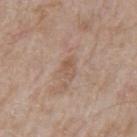biopsy_status: not biopsied; imaged during a skin examination
site: mid back
image:
  source: total-body photography crop
  field_of_view_mm: 15
patient:
  sex: male
  age_approx: 65